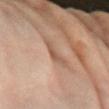notes = imaged on a skin check; not biopsied | acquisition = 15 mm crop, total-body photography | subject = female, aged around 65 | body site = the right forearm.